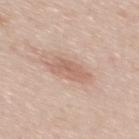  biopsy_status: not biopsied; imaged during a skin examination
  image:
    source: total-body photography crop
    field_of_view_mm: 15
  lesion_size:
    long_diameter_mm_approx: 4.0
  patient:
    sex: male
    age_approx: 35
  site: mid back